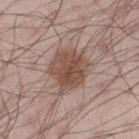Impression:
The lesion was tiled from a total-body skin photograph and was not biopsied.
Acquisition and patient details:
A male patient aged 28 to 32. Cropped from a whole-body photographic skin survey; the tile spans about 15 mm. The lesion is on the right thigh.